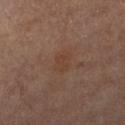workup — no biopsy performed (imaged during a skin exam) | illumination — cross-polarized | image-analysis metrics — a shape eccentricity near 0.65 and two-axis asymmetry of about 0.25; a lesion color around L≈38 a*≈18 b*≈26 in CIELAB, roughly 4 lightness units darker than nearby skin, and a normalized border contrast of about 5 | patient — male, in their mid-80s or older | image — total-body-photography crop, ~15 mm field of view | lesion diameter — about 2.5 mm | body site — the right lower leg.